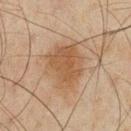Case summary:
* follow-up — total-body-photography surveillance lesion; no biopsy
* body site — the chest
* illumination — cross-polarized
* size — ≈5 mm
* patient — male, aged approximately 45
* image source — ~15 mm tile from a whole-body skin photo